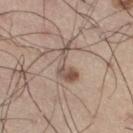From the right thigh. Imaged with white-light lighting. A male subject approximately 55 years of age. This image is a 15 mm lesion crop taken from a total-body photograph. About 4.5 mm across.